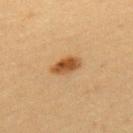Case summary:
• follow-up · catalogued during a skin exam; not biopsied
• body site · the arm
• size · about 3.5 mm
• image source · 15 mm crop, total-body photography
• lighting · cross-polarized
• subject · female, aged 38 to 42
• automated lesion analysis · a footprint of about 5.5 mm² and an outline eccentricity of about 0.85 (0 = round, 1 = elongated); a classifier nevus-likeness of about 100/100 and a lesion-detection confidence of about 100/100